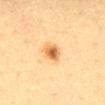acquisition: total-body-photography crop, ~15 mm field of view
anatomic site: the mid back
automated lesion analysis: a lesion area of about 5 mm², an outline eccentricity of about 0.5 (0 = round, 1 = elongated), and a shape-asymmetry score of about 0.2 (0 = symmetric); peripheral color asymmetry of about 1.5; a nevus-likeness score of about 95/100 and lesion-presence confidence of about 100/100
tile lighting: cross-polarized illumination
subject: female, approximately 40 years of age